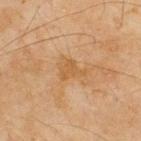Q: Is there a histopathology result?
A: imaged on a skin check; not biopsied
Q: What are the patient's age and sex?
A: male, approximately 45 years of age
Q: What kind of image is this?
A: ~15 mm crop, total-body skin-cancer survey
Q: How was the tile lit?
A: cross-polarized
Q: How large is the lesion?
A: ~3 mm (longest diameter)
Q: Where on the body is the lesion?
A: the upper back